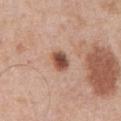Notes:
• notes: total-body-photography surveillance lesion; no biopsy
• body site: the abdomen
• tile lighting: white-light illumination
• image: ~15 mm crop, total-body skin-cancer survey
• subject: male, approximately 70 years of age